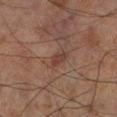Notes:
– workup · imaged on a skin check; not biopsied